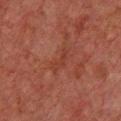Imaged during a routine full-body skin examination; the lesion was not biopsied and no histopathology is available. A male patient roughly 60 years of age. This image is a 15 mm lesion crop taken from a total-body photograph. The lesion is on the chest.